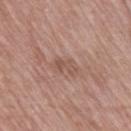{
  "biopsy_status": "not biopsied; imaged during a skin examination",
  "patient": {
    "sex": "female",
    "age_approx": 75
  },
  "lighting": "white-light",
  "lesion_size": {
    "long_diameter_mm_approx": 3.0
  },
  "image": {
    "source": "total-body photography crop",
    "field_of_view_mm": 15
  },
  "site": "right thigh"
}